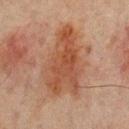Notes:
- follow-up: catalogued during a skin exam; not biopsied
- lesion diameter: ≈8.5 mm
- image source: ~15 mm tile from a whole-body skin photo
- patient: male, aged approximately 65
- illumination: cross-polarized illumination
- anatomic site: the mid back